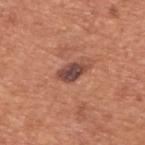Impression: No biopsy was performed on this lesion — it was imaged during a full skin examination and was not determined to be concerning. Image and clinical context: On the back. A 15 mm crop from a total-body photograph taken for skin-cancer surveillance. The lesion's longest dimension is about 3.5 mm. A male subject aged approximately 65. The total-body-photography lesion software estimated an outline eccentricity of about 0.85 (0 = round, 1 = elongated) and two-axis asymmetry of about 0.2. It also reported a lesion color around L≈45 a*≈22 b*≈25 in CIELAB, a lesion–skin lightness drop of about 14, and a lesion-to-skin contrast of about 11 (normalized; higher = more distinct). The analysis additionally found internal color variation of about 4 on a 0–10 scale. And it measured a nevus-likeness score of about 35/100 and a detector confidence of about 100 out of 100 that the crop contains a lesion. Imaged with white-light lighting.